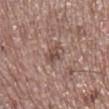{
  "biopsy_status": "not biopsied; imaged during a skin examination",
  "site": "right lower leg",
  "patient": {
    "sex": "male",
    "age_approx": 65
  },
  "image": {
    "source": "total-body photography crop",
    "field_of_view_mm": 15
  },
  "automated_metrics": {
    "border_irregularity_0_10": 2.5,
    "color_variation_0_10": 5.0,
    "peripheral_color_asymmetry": 2.0
  },
  "lighting": "white-light"
}